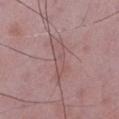Acquisition and patient details: A close-up tile cropped from a whole-body skin photograph, about 15 mm across. The tile uses white-light illumination. Automated tile analysis of the lesion measured a lesion area of about 9.5 mm² and an outline eccentricity of about 0.95 (0 = round, 1 = elongated). The analysis additionally found a lesion color around L≈53 a*≈20 b*≈19 in CIELAB, about 6 CIELAB-L* units darker than the surrounding skin, and a lesion-to-skin contrast of about 4.5 (normalized; higher = more distinct). It also reported border irregularity of about 6 on a 0–10 scale and a peripheral color-asymmetry measure near 1. And it measured a classifier nevus-likeness of about 0/100 and a lesion-detection confidence of about 80/100. From the left lower leg. The patient is a male approximately 50 years of age.